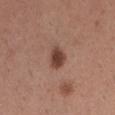Assessment:
This lesion was catalogued during total-body skin photography and was not selected for biopsy.
Clinical summary:
Captured under white-light illumination. From the right thigh. A female subject aged 28 to 32. A lesion tile, about 15 mm wide, cut from a 3D total-body photograph. Measured at roughly 3 mm in maximum diameter.